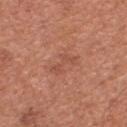• workup · total-body-photography surveillance lesion; no biopsy
• subject · male, about 65 years old
• imaging modality · 15 mm crop, total-body photography
• location · the front of the torso
• lesion size · ≈3.5 mm
• illumination · white-light illumination
• image-analysis metrics · an outline eccentricity of about 0.9 (0 = round, 1 = elongated) and a shape-asymmetry score of about 0.45 (0 = symmetric); about 6 CIELAB-L* units darker than the surrounding skin